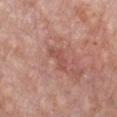Q: Patient demographics?
A: male, about 80 years old
Q: What is the anatomic site?
A: the front of the torso
Q: How was this image acquired?
A: 15 mm crop, total-body photography
Q: What is the lesion's diameter?
A: about 3.5 mm
Q: What did automated image analysis measure?
A: a lesion area of about 4 mm², an eccentricity of roughly 0.9, and two-axis asymmetry of about 0.5; a lesion color around L≈52 a*≈25 b*≈28 in CIELAB, a lesion–skin lightness drop of about 8, and a lesion-to-skin contrast of about 6 (normalized; higher = more distinct); a border-irregularity index near 5.5/10 and internal color variation of about 0 on a 0–10 scale